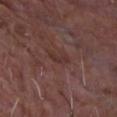Notes:
- location — the chest
- acquisition — total-body-photography crop, ~15 mm field of view
- subject — male, in their mid- to late 60s